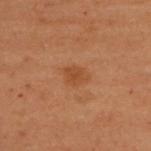{"biopsy_status": "not biopsied; imaged during a skin examination", "site": "back", "image": {"source": "total-body photography crop", "field_of_view_mm": 15}, "patient": {"sex": "female", "age_approx": 50}}